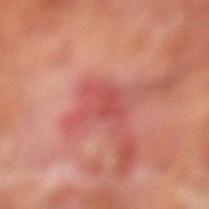The lesion was photographed on a routine skin check and not biopsied; there is no pathology result. A 15 mm close-up extracted from a 3D total-body photography capture. The patient is a male aged 68 to 72. The lesion is located on the left lower leg.